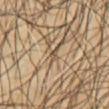Recorded during total-body skin imaging; not selected for excision or biopsy. A male patient, in their mid-40s. On the chest. The tile uses cross-polarized illumination. Measured at roughly 1.5 mm in maximum diameter. A 15 mm close-up tile from a total-body photography series done for melanoma screening.